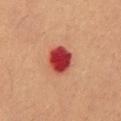Clinical impression: The lesion was tiled from a total-body skin photograph and was not biopsied. Image and clinical context: A region of skin cropped from a whole-body photographic capture, roughly 15 mm wide. The recorded lesion diameter is about 3.5 mm. The lesion is located on the abdomen. Imaged with cross-polarized lighting. A male patient, aged approximately 55. Automated image analysis of the tile measured an area of roughly 9 mm² and two-axis asymmetry of about 0.1. The software also gave a mean CIELAB color near L≈37 a*≈36 b*≈28, a lesion–skin lightness drop of about 17, and a normalized lesion–skin contrast near 13.5. And it measured a border-irregularity index near 1/10, internal color variation of about 5.5 on a 0–10 scale, and peripheral color asymmetry of about 2. The software also gave a classifier nevus-likeness of about 0/100.